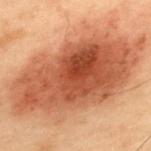– workup · imaged on a skin check; not biopsied
– patient · male, about 55 years old
– anatomic site · the upper back
– imaging modality · ~15 mm tile from a whole-body skin photo
– tile lighting · cross-polarized
– diameter · about 16.5 mm
– TBP lesion metrics · a footprint of about 95 mm², an outline eccentricity of about 0.85 (0 = round, 1 = elongated), and a symmetry-axis asymmetry near 0.15; an average lesion color of about L≈46 a*≈23 b*≈31 (CIELAB), roughly 14 lightness units darker than nearby skin, and a normalized lesion–skin contrast near 10; a classifier nevus-likeness of about 95/100 and a detector confidence of about 100 out of 100 that the crop contains a lesion